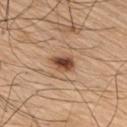Recorded during total-body skin imaging; not selected for excision or biopsy. On the arm. A male subject in their mid- to late 70s. A 15 mm close-up extracted from a 3D total-body photography capture.